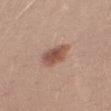biopsy_status: not biopsied; imaged during a skin examination
site: leg
lighting: white-light
patient:
  sex: female
  age_approx: 25
lesion_size:
  long_diameter_mm_approx: 4.0
image:
  source: total-body photography crop
  field_of_view_mm: 15
automated_metrics:
  area_mm2_approx: 8.0
  eccentricity: 0.8
  shape_asymmetry: 0.25
  cielab_L: 53
  cielab_a: 22
  cielab_b: 27
  vs_skin_darker_L: 12.0